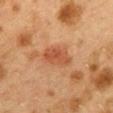Recorded during total-body skin imaging; not selected for excision or biopsy.
Captured under cross-polarized illumination.
A female subject, in their 40s.
Automated tile analysis of the lesion measured an outline eccentricity of about 0.85 (0 = round, 1 = elongated) and a symmetry-axis asymmetry near 0.25. The software also gave a mean CIELAB color near L≈43 a*≈23 b*≈32, a lesion–skin lightness drop of about 8, and a normalized lesion–skin contrast near 7.
On the mid back.
Cropped from a whole-body photographic skin survey; the tile spans about 15 mm.
About 4.5 mm across.